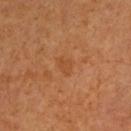notes: total-body-photography surveillance lesion; no biopsy
subject: male, aged approximately 60
body site: the arm
imaging modality: ~15 mm tile from a whole-body skin photo